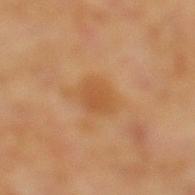Part of a total-body skin-imaging series; this lesion was reviewed on a skin check and was not flagged for biopsy. A male patient, about 60 years old. Imaged with cross-polarized lighting. Automated tile analysis of the lesion measured a lesion area of about 6 mm² and a shape-asymmetry score of about 0.2 (0 = symmetric). The analysis additionally found a border-irregularity index near 2/10 and radial color variation of about 0.5. The software also gave a lesion-detection confidence of about 100/100. The lesion is located on the left lower leg. About 3 mm across. A lesion tile, about 15 mm wide, cut from a 3D total-body photograph.